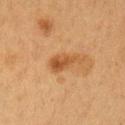| field | value |
|---|---|
| biopsy status | no biopsy performed (imaged during a skin exam) |
| patient | female, aged approximately 40 |
| anatomic site | the left upper arm |
| lesion size | ~3.5 mm (longest diameter) |
| imaging modality | ~15 mm tile from a whole-body skin photo |